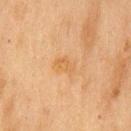biopsy status: catalogued during a skin exam; not biopsied | lesion size: ~2.5 mm (longest diameter) | image source: ~15 mm tile from a whole-body skin photo | subject: male, in their mid- to late 70s | lighting: cross-polarized | location: the chest | image-analysis metrics: an area of roughly 3.5 mm² and a shape-asymmetry score of about 0.2 (0 = symmetric); a border-irregularity rating of about 2/10, a within-lesion color-variation index near 1.5/10, and a peripheral color-asymmetry measure near 0.5.